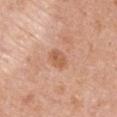Assessment:
Imaged during a routine full-body skin examination; the lesion was not biopsied and no histopathology is available.
Acquisition and patient details:
A close-up tile cropped from a whole-body skin photograph, about 15 mm across. A female subject aged 48–52. On the left upper arm.